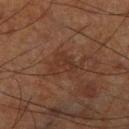tile lighting — cross-polarized illumination | body site — the right lower leg | subject — male, aged 68–72 | image source — total-body-photography crop, ~15 mm field of view.